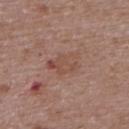Captured during whole-body skin photography for melanoma surveillance; the lesion was not biopsied. A female patient in their mid- to late 60s. The tile uses white-light illumination. This image is a 15 mm lesion crop taken from a total-body photograph. The recorded lesion diameter is about 4 mm. Located on the back.